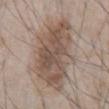Impression:
Recorded during total-body skin imaging; not selected for excision or biopsy.
Clinical summary:
This is a white-light tile. A male subject in their mid- to late 60s. The lesion's longest dimension is about 10 mm. A roughly 15 mm field-of-view crop from a total-body skin photograph. Automated tile analysis of the lesion measured a footprint of about 38 mm², an eccentricity of roughly 0.85, and a shape-asymmetry score of about 0.25 (0 = symmetric). It also reported an average lesion color of about L≈52 a*≈14 b*≈24 (CIELAB), about 11 CIELAB-L* units darker than the surrounding skin, and a lesion-to-skin contrast of about 8 (normalized; higher = more distinct). And it measured border irregularity of about 4 on a 0–10 scale, a color-variation rating of about 5/10, and radial color variation of about 2. The software also gave a nevus-likeness score of about 70/100 and a lesion-detection confidence of about 95/100. On the front of the torso.